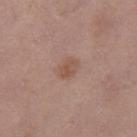Case summary:
* follow-up — catalogued during a skin exam; not biopsied
* patient — female, aged around 40
* body site — the right thigh
* diameter — ~2.5 mm (longest diameter)
* imaging modality — total-body-photography crop, ~15 mm field of view